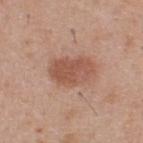{
  "biopsy_status": "not biopsied; imaged during a skin examination",
  "lesion_size": {
    "long_diameter_mm_approx": 5.0
  },
  "patient": {
    "sex": "male",
    "age_approx": 60
  },
  "image": {
    "source": "total-body photography crop",
    "field_of_view_mm": 15
  },
  "lighting": "white-light",
  "site": "upper back"
}